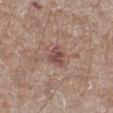{"biopsy_status": "not biopsied; imaged during a skin examination", "lesion_size": {"long_diameter_mm_approx": 2.5}, "patient": {"sex": "male", "age_approx": 65}, "site": "right lower leg", "automated_metrics": {"area_mm2_approx": 4.0, "shape_asymmetry": 0.3, "border_irregularity_0_10": 3.5, "color_variation_0_10": 2.0, "peripheral_color_asymmetry": 0.5, "nevus_likeness_0_100": 0, "lesion_detection_confidence_0_100": 100}, "image": {"source": "total-body photography crop", "field_of_view_mm": 15}}